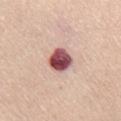Part of a total-body skin-imaging series; this lesion was reviewed on a skin check and was not flagged for biopsy.
The subject is a female aged approximately 70.
Approximately 3.5 mm at its widest.
A lesion tile, about 15 mm wide, cut from a 3D total-body photograph.
The lesion is on the front of the torso.